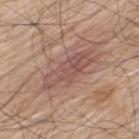Case summary:
* follow-up — no biopsy performed (imaged during a skin exam)
* image source — total-body-photography crop, ~15 mm field of view
* location — the upper back
* lesion size — ≈7 mm
* subject — male, aged around 70
* illumination — white-light illumination